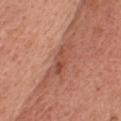biopsy status = no biopsy performed (imaged during a skin exam)
acquisition = ~15 mm crop, total-body skin-cancer survey
patient = female, roughly 50 years of age
location = the chest